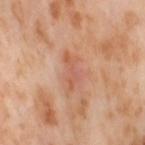The lesion was photographed on a routine skin check and not biopsied; there is no pathology result.
The recorded lesion diameter is about 4.5 mm.
This image is a 15 mm lesion crop taken from a total-body photograph.
The total-body-photography lesion software estimated a lesion area of about 7 mm², an outline eccentricity of about 0.9 (0 = round, 1 = elongated), and a symmetry-axis asymmetry near 0.35. And it measured a lesion color around L≈59 a*≈25 b*≈32 in CIELAB, about 7 CIELAB-L* units darker than the surrounding skin, and a lesion-to-skin contrast of about 5 (normalized; higher = more distinct). The analysis additionally found a border-irregularity index near 4.5/10, a within-lesion color-variation index near 2.5/10, and radial color variation of about 1. The software also gave a nevus-likeness score of about 0/100.
From the right thigh.
The patient is a female aged 53 to 57.